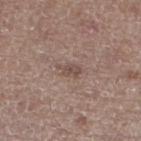site: the left lower leg; lesion diameter: ≈2.5 mm; subject: male, about 70 years old; acquisition: total-body-photography crop, ~15 mm field of view; illumination: white-light illumination.